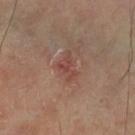Imaged during a routine full-body skin examination; the lesion was not biopsied and no histopathology is available.
The lesion is located on the right leg.
A female subject, aged approximately 60.
A close-up tile cropped from a whole-body skin photograph, about 15 mm across.